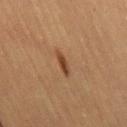Impression:
The lesion was photographed on a routine skin check and not biopsied; there is no pathology result.
Background:
A roughly 15 mm field-of-view crop from a total-body skin photograph. A female subject aged around 65. From the right thigh. The recorded lesion diameter is about 3.5 mm. This is a cross-polarized tile.